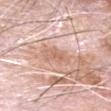notes: total-body-photography surveillance lesion; no biopsy | TBP lesion metrics: an average lesion color of about L≈65 a*≈21 b*≈29 (CIELAB) and roughly 8 lightness units darker than nearby skin; a classifier nevus-likeness of about 0/100 and lesion-presence confidence of about 80/100 | lesion diameter: about 3 mm | subject: male, aged 78 to 82 | site: the head or neck | acquisition: 15 mm crop, total-body photography.